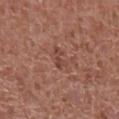Captured during whole-body skin photography for melanoma surveillance; the lesion was not biopsied.
This is a white-light tile.
From the abdomen.
The recorded lesion diameter is about 3 mm.
A roughly 15 mm field-of-view crop from a total-body skin photograph.
A male patient approximately 65 years of age.